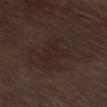  biopsy_status: not biopsied; imaged during a skin examination
  automated_metrics:
    eccentricity: 0.8
    shape_asymmetry: 0.65
    cielab_L: 22
    cielab_a: 14
    cielab_b: 15
    vs_skin_contrast_norm: 4.5
    border_irregularity_0_10: 7.5
    color_variation_0_10: 2.5
  lighting: white-light
  lesion_size:
    long_diameter_mm_approx: 4.0
  patient:
    sex: male
    age_approx: 70
  site: left lower leg
  image:
    source: total-body photography crop
    field_of_view_mm: 15